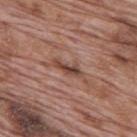This lesion was catalogued during total-body skin photography and was not selected for biopsy.
Cropped from a total-body skin-imaging series; the visible field is about 15 mm.
A male subject in their 70s.
About 3.5 mm across.
This is a white-light tile.
Located on the mid back.
Automated tile analysis of the lesion measured a footprint of about 4 mm², an eccentricity of roughly 0.95, and a shape-asymmetry score of about 0.3 (0 = symmetric). And it measured a nevus-likeness score of about 5/100 and a detector confidence of about 80 out of 100 that the crop contains a lesion.